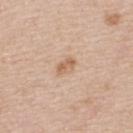Notes:
* follow-up · catalogued during a skin exam; not biopsied
* acquisition · ~15 mm tile from a whole-body skin photo
* site · the upper back
* illumination · white-light illumination
* lesion size · ~2.5 mm (longest diameter)
* patient · female, approximately 45 years of age